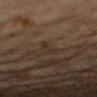image: ~15 mm tile from a whole-body skin photo
patient: female, roughly 60 years of age
diameter: ~2.5 mm (longest diameter)
automated lesion analysis: a mean CIELAB color near L≈31 a*≈15 b*≈24, roughly 5 lightness units darker than nearby skin, and a normalized border contrast of about 5.5; a border-irregularity rating of about 5.5/10 and radial color variation of about 0; an automated nevus-likeness rating near 0 out of 100
body site: the right forearm
lighting: cross-polarized illumination